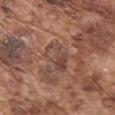Part of a total-body skin-imaging series; this lesion was reviewed on a skin check and was not flagged for biopsy. Located on the left upper arm. A 15 mm close-up tile from a total-body photography series done for melanoma screening. The patient is a male roughly 75 years of age. This is a white-light tile.